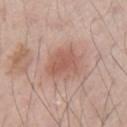notes: catalogued during a skin exam; not biopsied
site: the arm
image source: total-body-photography crop, ~15 mm field of view
subject: male, in their 70s
tile lighting: white-light
diameter: ~4 mm (longest diameter)